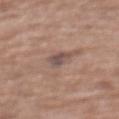workup — total-body-photography surveillance lesion; no biopsy
body site — the mid back
lighting — white-light illumination
automated lesion analysis — a lesion color around L≈50 a*≈16 b*≈21 in CIELAB, a lesion–skin lightness drop of about 9, and a lesion-to-skin contrast of about 7 (normalized; higher = more distinct); lesion-presence confidence of about 100/100
subject — female, roughly 75 years of age
lesion diameter — ~2.5 mm (longest diameter)
image source — ~15 mm tile from a whole-body skin photo